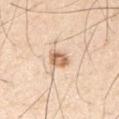The lesion was photographed on a routine skin check and not biopsied; there is no pathology result. This is a white-light tile. The lesion is located on the arm. Automated image analysis of the tile measured a footprint of about 4.5 mm² and two-axis asymmetry of about 0.25. A close-up tile cropped from a whole-body skin photograph, about 15 mm across. The patient is a male approximately 30 years of age. About 2.5 mm across.